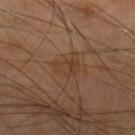Recorded during total-body skin imaging; not selected for excision or biopsy.
The subject is a male roughly 70 years of age.
The lesion is on the right lower leg.
A 15 mm crop from a total-body photograph taken for skin-cancer surveillance.
Imaged with cross-polarized lighting.